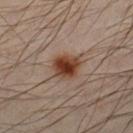Q: Was this lesion biopsied?
A: total-body-photography surveillance lesion; no biopsy
Q: What is the anatomic site?
A: the front of the torso
Q: What is the lesion's diameter?
A: about 3.5 mm
Q: How was the tile lit?
A: cross-polarized
Q: What are the patient's age and sex?
A: male, in their mid-30s
Q: What kind of image is this?
A: ~15 mm tile from a whole-body skin photo
Q: Automated lesion metrics?
A: a footprint of about 9 mm² and a shape eccentricity near 0.5